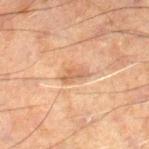| field | value |
|---|---|
| workup | total-body-photography surveillance lesion; no biopsy |
| body site | the leg |
| image | ~15 mm crop, total-body skin-cancer survey |
| subject | male, roughly 60 years of age |
| tile lighting | cross-polarized |
| lesion size | ~3 mm (longest diameter) |